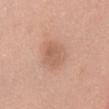notes — imaged on a skin check; not biopsied | location — the chest | subject — female, in their 50s | image — total-body-photography crop, ~15 mm field of view.